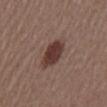A male subject, approximately 55 years of age.
The recorded lesion diameter is about 4 mm.
The tile uses white-light illumination.
Automated image analysis of the tile measured a mean CIELAB color near L≈37 a*≈19 b*≈22 and roughly 12 lightness units darker than nearby skin. The analysis additionally found a peripheral color-asymmetry measure near 0.5.
From the abdomen.
A 15 mm close-up tile from a total-body photography series done for melanoma screening.